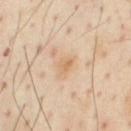<lesion>
  <biopsy_status>not biopsied; imaged during a skin examination</biopsy_status>
  <image>
    <source>total-body photography crop</source>
    <field_of_view_mm>15</field_of_view_mm>
  </image>
  <patient>
    <sex>male</sex>
    <age_approx>55</age_approx>
  </patient>
  <automated_metrics>
    <border_irregularity_0_10>3.5</border_irregularity_0_10>
    <color_variation_0_10>3.0</color_variation_0_10>
    <peripheral_color_asymmetry>1.0</peripheral_color_asymmetry>
    <nevus_likeness_0_100>20</nevus_likeness_0_100>
    <lesion_detection_confidence_0_100>100</lesion_detection_confidence_0_100>
  </automated_metrics>
  <site>front of the torso</site>
  <lesion_size>
    <long_diameter_mm_approx>3.0</long_diameter_mm_approx>
  </lesion_size>
  <lighting>cross-polarized</lighting>
</lesion>